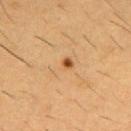Q: Was this lesion biopsied?
A: imaged on a skin check; not biopsied
Q: What are the patient's age and sex?
A: male, aged 53–57
Q: What kind of image is this?
A: ~15 mm crop, total-body skin-cancer survey
Q: Illumination type?
A: cross-polarized
Q: What is the lesion's diameter?
A: about 4 mm
Q: What is the anatomic site?
A: the chest